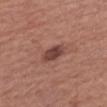{"biopsy_status": "not biopsied; imaged during a skin examination", "site": "right forearm", "automated_metrics": {"area_mm2_approx": 5.5, "eccentricity": 0.7, "shape_asymmetry": 0.25, "border_irregularity_0_10": 2.0, "color_variation_0_10": 3.5, "nevus_likeness_0_100": 80}, "patient": {"sex": "female", "age_approx": 60}, "lesion_size": {"long_diameter_mm_approx": 3.0}, "image": {"source": "total-body photography crop", "field_of_view_mm": 15}}